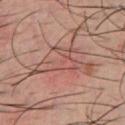Clinical impression: Imaged during a routine full-body skin examination; the lesion was not biopsied and no histopathology is available. Acquisition and patient details: A male subject approximately 40 years of age. A 15 mm close-up tile from a total-body photography series done for melanoma screening. The lesion is located on the front of the torso.